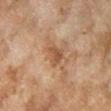Recorded during total-body skin imaging; not selected for excision or biopsy. On the leg. Approximately 3 mm at its widest. An algorithmic analysis of the crop reported a lesion color around L≈45 a*≈18 b*≈30 in CIELAB and a lesion–skin lightness drop of about 9. It also reported border irregularity of about 3.5 on a 0–10 scale, a within-lesion color-variation index near 2.5/10, and a peripheral color-asymmetry measure near 1. A 15 mm crop from a total-body photograph taken for skin-cancer surveillance. The subject is a female roughly 60 years of age.